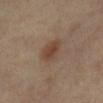Q: Was this lesion biopsied?
A: imaged on a skin check; not biopsied
Q: How was the tile lit?
A: cross-polarized illumination
Q: What are the patient's age and sex?
A: female, aged approximately 65
Q: Where on the body is the lesion?
A: the right lower leg
Q: How large is the lesion?
A: ~3 mm (longest diameter)
Q: How was this image acquired?
A: 15 mm crop, total-body photography